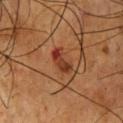The lesion was tiled from a total-body skin photograph and was not biopsied. The recorded lesion diameter is about 3 mm. A 15 mm close-up extracted from a 3D total-body photography capture. A male patient aged 53–57. Automated tile analysis of the lesion measured a footprint of about 4.5 mm², an outline eccentricity of about 0.9 (0 = round, 1 = elongated), and two-axis asymmetry of about 0.35. The software also gave an average lesion color of about L≈34 a*≈26 b*≈31 (CIELAB), about 10 CIELAB-L* units darker than the surrounding skin, and a normalized lesion–skin contrast near 9. The lesion is on the chest.